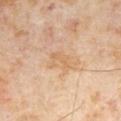<record>
<biopsy_status>not biopsied; imaged during a skin examination</biopsy_status>
<image>
  <source>total-body photography crop</source>
  <field_of_view_mm>15</field_of_view_mm>
</image>
<automated_metrics>
  <area_mm2_approx>4.5</area_mm2_approx>
  <eccentricity>0.8</eccentricity>
  <shape_asymmetry>0.45</shape_asymmetry>
  <cielab_L>64</cielab_L>
  <cielab_a>18</cielab_a>
  <cielab_b>36</cielab_b>
  <vs_skin_contrast_norm>5.0</vs_skin_contrast_norm>
</automated_metrics>
<patient>
  <sex>male</sex>
  <age_approx>65</age_approx>
</patient>
</record>